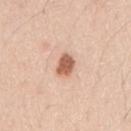{"biopsy_status": "not biopsied; imaged during a skin examination", "image": {"source": "total-body photography crop", "field_of_view_mm": 15}, "patient": {"sex": "male", "age_approx": 30}, "automated_metrics": {"area_mm2_approx": 5.0, "shape_asymmetry": 0.2, "border_irregularity_0_10": 1.5, "color_variation_0_10": 2.0, "peripheral_color_asymmetry": 0.5}, "site": "right upper arm", "lesion_size": {"long_diameter_mm_approx": 3.0}, "lighting": "white-light"}